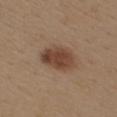workup = catalogued during a skin exam; not biopsied | tile lighting = white-light | anatomic site = the upper back | lesion size = about 5 mm | patient = female, aged around 40 | image = 15 mm crop, total-body photography.